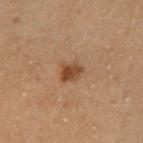Clinical impression:
Imaged during a routine full-body skin examination; the lesion was not biopsied and no histopathology is available.
Acquisition and patient details:
The lesion is located on the left lower leg. A male patient, aged 68–72. The lesion's longest dimension is about 2.5 mm. This is a cross-polarized tile. A 15 mm crop from a total-body photograph taken for skin-cancer surveillance.